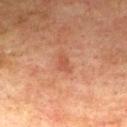The lesion's longest dimension is about 2.5 mm.
A male patient, in their mid- to late 70s.
Imaged with cross-polarized lighting.
Automated image analysis of the tile measured a footprint of about 3 mm² and an outline eccentricity of about 0.8 (0 = round, 1 = elongated). And it measured a border-irregularity rating of about 3.5/10, internal color variation of about 1 on a 0–10 scale, and a peripheral color-asymmetry measure near 0.5. The software also gave a nevus-likeness score of about 5/100 and a detector confidence of about 100 out of 100 that the crop contains a lesion.
A roughly 15 mm field-of-view crop from a total-body skin photograph.
The lesion is located on the mid back.